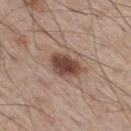Clinical impression: The lesion was photographed on a routine skin check and not biopsied; there is no pathology result. Background: Captured under white-light illumination. A roughly 15 mm field-of-view crop from a total-body skin photograph. On the right thigh. A male patient, aged approximately 60.